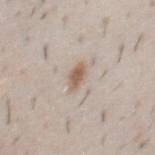Recorded during total-body skin imaging; not selected for excision or biopsy.